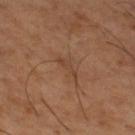workup: catalogued during a skin exam; not biopsied | image source: ~15 mm tile from a whole-body skin photo | body site: the right thigh | patient: male, aged around 65 | illumination: cross-polarized | automated metrics: an area of roughly 3 mm², a shape eccentricity near 0.95, and two-axis asymmetry of about 0.45; an average lesion color of about L≈42 a*≈20 b*≈31 (CIELAB), a lesion–skin lightness drop of about 6, and a normalized lesion–skin contrast near 5.